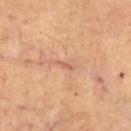biopsy status: no biopsy performed (imaged during a skin exam) | illumination: cross-polarized | patient: male, aged 63–67 | body site: the back | image: ~15 mm tile from a whole-body skin photo | TBP lesion metrics: an area of roughly 2 mm² and an outline eccentricity of about 0.95 (0 = round, 1 = elongated) | size: ~2.5 mm (longest diameter).